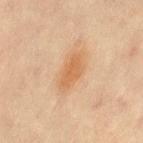Case summary:
* follow-up · no biopsy performed (imaged during a skin exam)
* automated lesion analysis · a lesion color around L≈55 a*≈19 b*≈35 in CIELAB and a normalized border contrast of about 6.5; an automated nevus-likeness rating near 90 out of 100 and lesion-presence confidence of about 100/100
* acquisition · total-body-photography crop, ~15 mm field of view
* lighting · cross-polarized
* size · ~4.5 mm (longest diameter)
* location · the left thigh
* patient · female, roughly 45 years of age